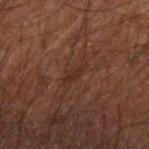The lesion was photographed on a routine skin check and not biopsied; there is no pathology result.
Located on the left forearm.
Automated image analysis of the tile measured a normalized border contrast of about 6. The analysis additionally found a border-irregularity rating of about 4/10, internal color variation of about 0 on a 0–10 scale, and radial color variation of about 0. The software also gave a classifier nevus-likeness of about 0/100 and a detector confidence of about 55 out of 100 that the crop contains a lesion.
The subject is a male in their 50s.
Longest diameter approximately 2.5 mm.
A 15 mm close-up tile from a total-body photography series done for melanoma screening.